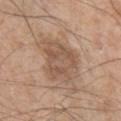No biopsy was performed on this lesion — it was imaged during a full skin examination and was not determined to be concerning. The total-body-photography lesion software estimated an eccentricity of roughly 0.65 and a shape-asymmetry score of about 0.3 (0 = symmetric). And it measured a mean CIELAB color near L≈54 a*≈17 b*≈29 and about 9 CIELAB-L* units darker than the surrounding skin. It also reported a lesion-detection confidence of about 100/100. A male subject, aged approximately 55. Located on the chest. Measured at roughly 5.5 mm in maximum diameter. A roughly 15 mm field-of-view crop from a total-body skin photograph.